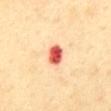Clinical impression: This lesion was catalogued during total-body skin photography and was not selected for biopsy.